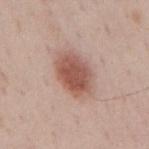Part of a total-body skin-imaging series; this lesion was reviewed on a skin check and was not flagged for biopsy. An algorithmic analysis of the crop reported an area of roughly 15 mm², an eccentricity of roughly 0.75, and two-axis asymmetry of about 0.1. The analysis additionally found a lesion color around L≈55 a*≈22 b*≈26 in CIELAB, a lesion–skin lightness drop of about 13, and a normalized border contrast of about 9. The software also gave a classifier nevus-likeness of about 100/100. A region of skin cropped from a whole-body photographic capture, roughly 15 mm wide. The subject is a male roughly 70 years of age. On the mid back. The lesion's longest dimension is about 5.5 mm.